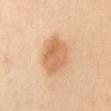biopsy status = total-body-photography surveillance lesion; no biopsy | illumination = cross-polarized illumination | site = the mid back | lesion diameter = ~5.5 mm (longest diameter) | image source = 15 mm crop, total-body photography | patient = female, in their mid- to late 40s.